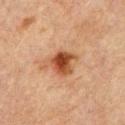Impression:
The lesion was photographed on a routine skin check and not biopsied; there is no pathology result.
Context:
Automated image analysis of the tile measured a lesion color around L≈40 a*≈22 b*≈31 in CIELAB, about 12 CIELAB-L* units darker than the surrounding skin, and a normalized lesion–skin contrast near 10. The lesion is located on the upper back. The patient is a male in their mid-60s. Captured under cross-polarized illumination. A 15 mm close-up tile from a total-body photography series done for melanoma screening.